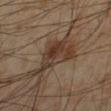biopsy_status: not biopsied; imaged during a skin examination
image:
  source: total-body photography crop
  field_of_view_mm: 15
patient:
  sex: male
  age_approx: 55
lighting: cross-polarized
site: leg
automated_metrics:
  vs_skin_darker_L: 10.0
  vs_skin_contrast_norm: 8.5
  border_irregularity_0_10: 6.5
  color_variation_0_10: 5.5
  peripheral_color_asymmetry: 1.5
  nevus_likeness_0_100: 95
  lesion_detection_confidence_0_100: 90